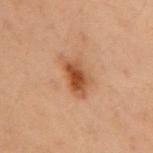biopsy status: no biopsy performed (imaged during a skin exam) | location: the arm | image source: 15 mm crop, total-body photography | patient: female, aged 38 to 42.